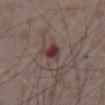Assessment:
Imaged during a routine full-body skin examination; the lesion was not biopsied and no histopathology is available.
Background:
A lesion tile, about 15 mm wide, cut from a 3D total-body photograph. The patient is a male about 75 years old. Located on the abdomen.